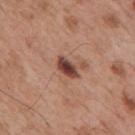Imaged during a routine full-body skin examination; the lesion was not biopsied and no histopathology is available. On the mid back. The patient is a male approximately 55 years of age. Automated tile analysis of the lesion measured a lesion area of about 4.5 mm² and two-axis asymmetry of about 0.2. It also reported a mean CIELAB color near L≈43 a*≈22 b*≈25 and a normalized border contrast of about 12. The software also gave a classifier nevus-likeness of about 30/100 and lesion-presence confidence of about 100/100. The lesion's longest dimension is about 3 mm. A 15 mm close-up extracted from a 3D total-body photography capture.